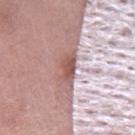patient: female, aged 68–72; diameter: about 3.5 mm; automated metrics: an automated nevus-likeness rating near 0 out of 100 and a lesion-detection confidence of about 90/100; acquisition: ~15 mm crop, total-body skin-cancer survey; site: the right thigh.